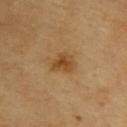{
  "biopsy_status": "not biopsied; imaged during a skin examination",
  "image": {
    "source": "total-body photography crop",
    "field_of_view_mm": 15
  },
  "patient": {
    "sex": "male",
    "age_approx": 60
  },
  "lighting": "cross-polarized",
  "site": "upper back",
  "lesion_size": {
    "long_diameter_mm_approx": 3.0
  },
  "automated_metrics": {
    "area_mm2_approx": 5.5,
    "shape_asymmetry": 0.35,
    "vs_skin_darker_L": 9.0,
    "vs_skin_contrast_norm": 7.5,
    "nevus_likeness_0_100": 90,
    "lesion_detection_confidence_0_100": 100
  }
}